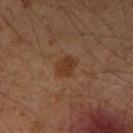- workup — total-body-photography surveillance lesion; no biopsy
- body site — the right upper arm
- size — ~3 mm (longest diameter)
- imaging modality — ~15 mm crop, total-body skin-cancer survey
- tile lighting — cross-polarized illumination
- patient — male, about 50 years old